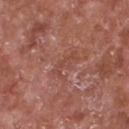Context: The tile uses white-light illumination. A male patient, roughly 65 years of age. An algorithmic analysis of the crop reported a color-variation rating of about 0/10 and a peripheral color-asymmetry measure near 0. The analysis additionally found an automated nevus-likeness rating near 0 out of 100 and a detector confidence of about 55 out of 100 that the crop contains a lesion. The recorded lesion diameter is about 3.5 mm. A close-up tile cropped from a whole-body skin photograph, about 15 mm across. Located on the chest.